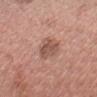This lesion was catalogued during total-body skin photography and was not selected for biopsy.
Measured at roughly 4.5 mm in maximum diameter.
The total-body-photography lesion software estimated a shape eccentricity near 0.85 and a symmetry-axis asymmetry near 0.2. And it measured a border-irregularity index near 2.5/10, a color-variation rating of about 3.5/10, and peripheral color asymmetry of about 1.
A lesion tile, about 15 mm wide, cut from a 3D total-body photograph.
This is a white-light tile.
The subject is a male roughly 30 years of age.